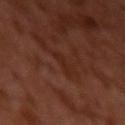{"biopsy_status": "not biopsied; imaged during a skin examination"}